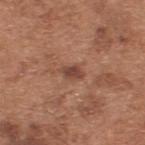The lesion was photographed on a routine skin check and not biopsied; there is no pathology result. Captured under white-light illumination. The lesion is located on the back. An algorithmic analysis of the crop reported a shape-asymmetry score of about 0.2 (0 = symmetric). It also reported a mean CIELAB color near L≈44 a*≈22 b*≈27, a lesion–skin lightness drop of about 10, and a normalized border contrast of about 8. The software also gave lesion-presence confidence of about 100/100. The subject is a male in their mid-60s. This image is a 15 mm lesion crop taken from a total-body photograph. About 2.5 mm across.